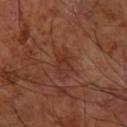Recorded during total-body skin imaging; not selected for excision or biopsy. The patient is a male aged 58 to 62. A 15 mm close-up extracted from a 3D total-body photography capture. From the right forearm.